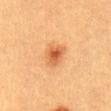body site: the abdomen
lesion size: ~3 mm (longest diameter)
subject: female, aged approximately 40
automated lesion analysis: an outline eccentricity of about 0.6 (0 = round, 1 = elongated) and two-axis asymmetry of about 0.25; a border-irregularity rating of about 2.5/10 and radial color variation of about 1.5
acquisition: total-body-photography crop, ~15 mm field of view